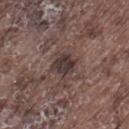Captured during whole-body skin photography for melanoma surveillance; the lesion was not biopsied.
The lesion is located on the leg.
Captured under white-light illumination.
Longest diameter approximately 2.5 mm.
The total-body-photography lesion software estimated a border-irregularity index near 2.5/10 and a within-lesion color-variation index near 2.5/10.
The subject is a male aged approximately 75.
A lesion tile, about 15 mm wide, cut from a 3D total-body photograph.